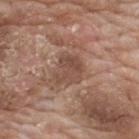Q: Is there a histopathology result?
A: no biopsy performed (imaged during a skin exam)
Q: What is the anatomic site?
A: the back
Q: Lesion size?
A: ~4 mm (longest diameter)
Q: Automated lesion metrics?
A: a border-irregularity index near 4/10 and peripheral color asymmetry of about 1
Q: Patient demographics?
A: male, aged approximately 60
Q: Illumination type?
A: white-light illumination
Q: How was this image acquired?
A: 15 mm crop, total-body photography